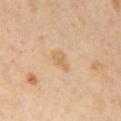Imaged during a routine full-body skin examination; the lesion was not biopsied and no histopathology is available. This is a cross-polarized tile. A female subject, about 40 years old. A lesion tile, about 15 mm wide, cut from a 3D total-body photograph. On the left arm. About 3 mm across. Automated tile analysis of the lesion measured a footprint of about 3.5 mm², an eccentricity of roughly 0.8, and two-axis asymmetry of about 0.3. And it measured an average lesion color of about L≈67 a*≈17 b*≈39 (CIELAB), about 7 CIELAB-L* units darker than the surrounding skin, and a normalized lesion–skin contrast near 6. And it measured a border-irregularity index near 3/10 and a color-variation rating of about 1.5/10.